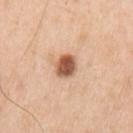follow-up: catalogued during a skin exam; not biopsied
anatomic site: the left upper arm
lighting: white-light
size: ≈3 mm
automated lesion analysis: a border-irregularity index near 1.5/10 and a within-lesion color-variation index near 5/10; a classifier nevus-likeness of about 100/100 and a lesion-detection confidence of about 100/100
image: ~15 mm crop, total-body skin-cancer survey
patient: male, in their 70s